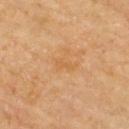Imaged during a routine full-body skin examination; the lesion was not biopsied and no histopathology is available.
Imaged with cross-polarized lighting.
About 2.5 mm across.
Automated image analysis of the tile measured a classifier nevus-likeness of about 0/100 and lesion-presence confidence of about 100/100.
A 15 mm close-up extracted from a 3D total-body photography capture.
A female patient, aged around 70.
From the upper back.